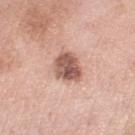workup: catalogued during a skin exam; not biopsied
body site: the leg
lighting: white-light
image source: total-body-photography crop, ~15 mm field of view
size: about 4 mm
patient: female, aged approximately 55